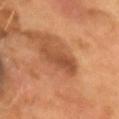Q: What is the imaging modality?
A: ~15 mm crop, total-body skin-cancer survey
Q: Where on the body is the lesion?
A: the head or neck
Q: What are the patient's age and sex?
A: male, aged approximately 55
Q: Illumination type?
A: cross-polarized
Q: What is the lesion's diameter?
A: ~4.5 mm (longest diameter)